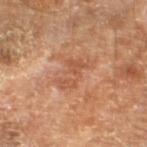follow-up: no biopsy performed (imaged during a skin exam) | subject: male, aged 68 to 72 | anatomic site: the left lower leg | image source: total-body-photography crop, ~15 mm field of view.